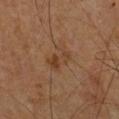Q: How was the tile lit?
A: cross-polarized
Q: Patient demographics?
A: male, aged 48–52
Q: What is the lesion's diameter?
A: ~3.5 mm (longest diameter)
Q: Automated lesion metrics?
A: a shape eccentricity near 0.75; a nevus-likeness score of about 40/100 and a detector confidence of about 100 out of 100 that the crop contains a lesion
Q: What is the anatomic site?
A: the chest
Q: How was this image acquired?
A: ~15 mm tile from a whole-body skin photo